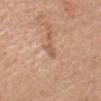The lesion was photographed on a routine skin check and not biopsied; there is no pathology result.
About 2 mm across.
Cropped from a whole-body photographic skin survey; the tile spans about 15 mm.
The patient is a female in their mid- to late 60s.
The lesion is on the head or neck.
Captured under white-light illumination.